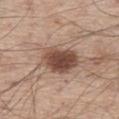<tbp_lesion>
<biopsy_status>not biopsied; imaged during a skin examination</biopsy_status>
<patient>
  <sex>male</sex>
  <age_approx>60</age_approx>
</patient>
<image>
  <source>total-body photography crop</source>
  <field_of_view_mm>15</field_of_view_mm>
</image>
<site>leg</site>
<automated_metrics>
  <cielab_L>49</cielab_L>
  <cielab_a>19</cielab_a>
  <cielab_b>26</cielab_b>
  <vs_skin_darker_L>14.0</vs_skin_darker_L>
  <vs_skin_contrast_norm>10.0</vs_skin_contrast_norm>
</automated_metrics>
</tbp_lesion>